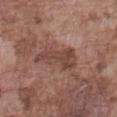• location · the front of the torso
• patient · male, in their mid- to late 70s
• lesion size · about 5.5 mm
• lighting · white-light
• acquisition · total-body-photography crop, ~15 mm field of view